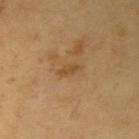Case summary:
- image source · total-body-photography crop, ~15 mm field of view
- lesion diameter · ~3 mm (longest diameter)
- patient · male, in their mid- to late 60s
- tile lighting · cross-polarized
- body site · the right upper arm